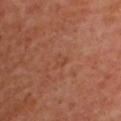  biopsy_status: not biopsied; imaged during a skin examination
  lighting: cross-polarized
  site: upper back
  patient:
    sex: female
    age_approx: 60
  image:
    source: total-body photography crop
    field_of_view_mm: 15
  automated_metrics:
    area_mm2_approx: 2.5
    eccentricity: 0.45
    shape_asymmetry: 0.35
    border_irregularity_0_10: 3.5
    peripheral_color_asymmetry: 0.0
    lesion_detection_confidence_0_100: 100
  lesion_size:
    long_diameter_mm_approx: 2.0